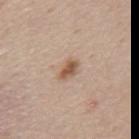No biopsy was performed on this lesion — it was imaged during a full skin examination and was not determined to be concerning.
Approximately 3 mm at its widest.
Captured under white-light illumination.
A male subject approximately 45 years of age.
Cropped from a whole-body photographic skin survey; the tile spans about 15 mm.
Automated tile analysis of the lesion measured a lesion area of about 4 mm², an outline eccentricity of about 0.8 (0 = round, 1 = elongated), and two-axis asymmetry of about 0.3. The analysis additionally found a lesion–skin lightness drop of about 12 and a normalized lesion–skin contrast near 8.5. And it measured an automated nevus-likeness rating near 90 out of 100 and lesion-presence confidence of about 100/100.
Located on the mid back.